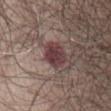No biopsy was performed on this lesion — it was imaged during a full skin examination and was not determined to be concerning.
A male subject aged around 80.
About 3.5 mm across.
Located on the abdomen.
A 15 mm crop from a total-body photograph taken for skin-cancer surveillance.